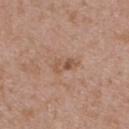This lesion was catalogued during total-body skin photography and was not selected for biopsy.
A female subject, aged approximately 30.
From the upper back.
This image is a 15 mm lesion crop taken from a total-body photograph.